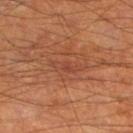notes — catalogued during a skin exam; not biopsied